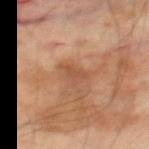body site: the right thigh; imaging modality: ~15 mm crop, total-body skin-cancer survey; patient: male, about 70 years old.